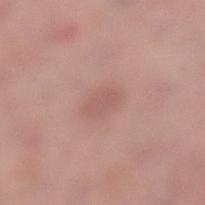Impression: This lesion was catalogued during total-body skin photography and was not selected for biopsy. Background: Captured under white-light illumination. The lesion is located on the left lower leg. A close-up tile cropped from a whole-body skin photograph, about 15 mm across. The patient is a female approximately 55 years of age.